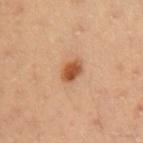workup = total-body-photography surveillance lesion; no biopsy
body site = the left upper arm
acquisition = ~15 mm crop, total-body skin-cancer survey
subject = female, approximately 35 years of age
lighting = cross-polarized illumination
lesion size = ≈2.5 mm